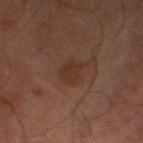{"biopsy_status": "not biopsied; imaged during a skin examination", "image": {"source": "total-body photography crop", "field_of_view_mm": 15}, "lighting": "cross-polarized", "patient": {"sex": "male", "age_approx": 70}, "lesion_size": {"long_diameter_mm_approx": 3.5}, "automated_metrics": {"area_mm2_approx": 5.5, "eccentricity": 0.8, "shape_asymmetry": 0.25, "color_variation_0_10": 1.5, "nevus_likeness_0_100": 60, "lesion_detection_confidence_0_100": 100}, "site": "left lower leg"}